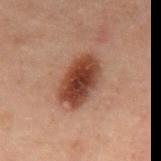Impression:
Captured during whole-body skin photography for melanoma surveillance; the lesion was not biopsied.
Acquisition and patient details:
Approximately 5.5 mm at its widest. Captured under cross-polarized illumination. A male subject aged around 60. Automated image analysis of the tile measured a lesion area of about 15 mm² and a shape-asymmetry score of about 0.15 (0 = symmetric). On the back. A close-up tile cropped from a whole-body skin photograph, about 15 mm across.